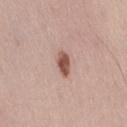{
  "biopsy_status": "not biopsied; imaged during a skin examination",
  "patient": {
    "sex": "male",
    "age_approx": 55
  },
  "site": "back",
  "automated_metrics": {
    "border_irregularity_0_10": 2.0,
    "color_variation_0_10": 2.5,
    "peripheral_color_asymmetry": 1.0,
    "nevus_likeness_0_100": 100,
    "lesion_detection_confidence_0_100": 100
  },
  "lighting": "white-light",
  "lesion_size": {
    "long_diameter_mm_approx": 3.0
  },
  "image": {
    "source": "total-body photography crop",
    "field_of_view_mm": 15
  }
}